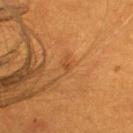The lesion was photographed on a routine skin check and not biopsied; there is no pathology result.
A 15 mm close-up tile from a total-body photography series done for melanoma screening.
Captured under cross-polarized illumination.
Located on the upper back.
The total-body-photography lesion software estimated a lesion color around L≈44 a*≈25 b*≈40 in CIELAB, a lesion–skin lightness drop of about 7, and a normalized lesion–skin contrast near 5.5. The analysis additionally found a border-irregularity index near 2.5/10, internal color variation of about 0 on a 0–10 scale, and a peripheral color-asymmetry measure near 0.
The patient is a female aged 28 to 32.